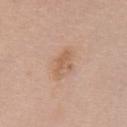biopsy status: total-body-photography surveillance lesion; no biopsy
TBP lesion metrics: border irregularity of about 3.5 on a 0–10 scale, a color-variation rating of about 2/10, and a peripheral color-asymmetry measure near 0.5; an automated nevus-likeness rating near 0 out of 100
imaging modality: total-body-photography crop, ~15 mm field of view
lesion diameter: ≈3 mm
location: the chest
patient: female, roughly 60 years of age